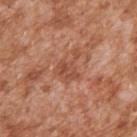  biopsy_status: not biopsied; imaged during a skin examination
  image:
    source: total-body photography crop
    field_of_view_mm: 15
  patient:
    sex: male
    age_approx: 45
  site: right upper arm